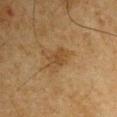Imaged during a routine full-body skin examination; the lesion was not biopsied and no histopathology is available.
The subject is a male aged 63–67.
About 3 mm across.
The tile uses cross-polarized illumination.
A region of skin cropped from a whole-body photographic capture, roughly 15 mm wide.
Located on the left upper arm.
The lesion-visualizer software estimated a mean CIELAB color near L≈37 a*≈15 b*≈31, roughly 6 lightness units darker than nearby skin, and a normalized lesion–skin contrast near 5.5. The analysis additionally found a classifier nevus-likeness of about 10/100 and a lesion-detection confidence of about 100/100.